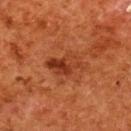– follow-up: no biopsy performed (imaged during a skin exam)
– lighting: cross-polarized
– image: total-body-photography crop, ~15 mm field of view
– size: ≈4.5 mm
– location: the upper back
– patient: female, aged 48 to 52